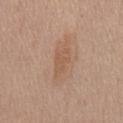Q: Was this lesion biopsied?
A: no biopsy performed (imaged during a skin exam)
Q: What did automated image analysis measure?
A: a nevus-likeness score of about 0/100 and a lesion-detection confidence of about 100/100
Q: How large is the lesion?
A: ≈4 mm
Q: How was the tile lit?
A: white-light
Q: How was this image acquired?
A: ~15 mm tile from a whole-body skin photo
Q: Where on the body is the lesion?
A: the chest
Q: What are the patient's age and sex?
A: male, aged around 55